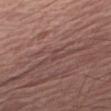Assessment:
The lesion was photographed on a routine skin check and not biopsied; there is no pathology result.